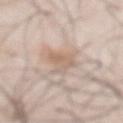Impression:
Recorded during total-body skin imaging; not selected for excision or biopsy.
Context:
Measured at roughly 4 mm in maximum diameter. This is a white-light tile. The subject is a male in their mid-50s. Automated tile analysis of the lesion measured a lesion area of about 7 mm², an outline eccentricity of about 0.75 (0 = round, 1 = elongated), and two-axis asymmetry of about 0.4. It also reported a lesion color around L≈64 a*≈15 b*≈27 in CIELAB and a lesion–skin lightness drop of about 9. Located on the abdomen. A 15 mm close-up extracted from a 3D total-body photography capture.